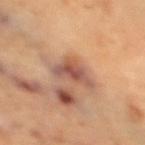Impression: Captured during whole-body skin photography for melanoma surveillance; the lesion was not biopsied. Clinical summary: Measured at roughly 5 mm in maximum diameter. The lesion-visualizer software estimated an area of roughly 8.5 mm² and two-axis asymmetry of about 0.45. And it measured a classifier nevus-likeness of about 5/100 and a detector confidence of about 100 out of 100 that the crop contains a lesion. This is a cross-polarized tile. A female subject approximately 70 years of age. A roughly 15 mm field-of-view crop from a total-body skin photograph. The lesion is located on the leg.